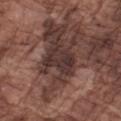Captured during whole-body skin photography for melanoma surveillance; the lesion was not biopsied.
This image is a 15 mm lesion crop taken from a total-body photograph.
Located on the left forearm.
A male subject, aged approximately 75.
Approximately 3.5 mm at its widest.
Automated image analysis of the tile measured a lesion color around L≈32 a*≈17 b*≈18 in CIELAB, a lesion–skin lightness drop of about 9, and a lesion-to-skin contrast of about 9.5 (normalized; higher = more distinct).
The tile uses white-light illumination.